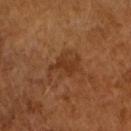The lesion was photographed on a routine skin check and not biopsied; there is no pathology result. A male subject aged 63–67. The lesion-visualizer software estimated a lesion area of about 8.5 mm², an outline eccentricity of about 0.7 (0 = round, 1 = elongated), and two-axis asymmetry of about 0.45. The lesion's longest dimension is about 4.5 mm. A region of skin cropped from a whole-body photographic capture, roughly 15 mm wide.